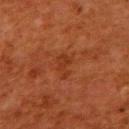This lesion was catalogued during total-body skin photography and was not selected for biopsy. A roughly 15 mm field-of-view crop from a total-body skin photograph. Located on the upper back. A female patient, roughly 50 years of age. This is a cross-polarized tile. The lesion-visualizer software estimated an area of roughly 4 mm², an outline eccentricity of about 0.85 (0 = round, 1 = elongated), and a shape-asymmetry score of about 0.5 (0 = symmetric). It also reported a border-irregularity index near 5.5/10, internal color variation of about 1 on a 0–10 scale, and a peripheral color-asymmetry measure near 0.5. The recorded lesion diameter is about 3 mm.